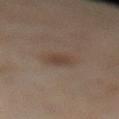{
  "biopsy_status": "not biopsied; imaged during a skin examination",
  "image": {
    "source": "total-body photography crop",
    "field_of_view_mm": 15
  },
  "patient": {
    "sex": "female",
    "age_approx": 50
  },
  "site": "right lower leg",
  "lighting": "cross-polarized",
  "lesion_size": {
    "long_diameter_mm_approx": 2.5
  }
}